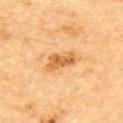follow-up: total-body-photography surveillance lesion; no biopsy
image: 15 mm crop, total-body photography
subject: male, in their mid- to late 80s or older
location: the upper back